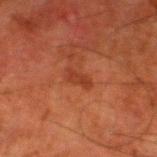Cropped from a total-body skin-imaging series; the visible field is about 15 mm.
From the left lower leg.
A male patient about 80 years old.